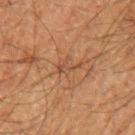Clinical impression:
No biopsy was performed on this lesion — it was imaged during a full skin examination and was not determined to be concerning.
Image and clinical context:
Automated image analysis of the tile measured a footprint of about 2.5 mm², an outline eccentricity of about 0.85 (0 = round, 1 = elongated), and two-axis asymmetry of about 0.55. The software also gave an automated nevus-likeness rating near 0 out of 100 and a detector confidence of about 60 out of 100 that the crop contains a lesion. Imaged with cross-polarized lighting. Cropped from a total-body skin-imaging series; the visible field is about 15 mm. On the left upper arm. The recorded lesion diameter is about 3 mm. A male patient, in their mid-60s.